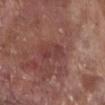Clinical impression:
This lesion was catalogued during total-body skin photography and was not selected for biopsy.
Image and clinical context:
A 15 mm close-up tile from a total-body photography series done for melanoma screening. The patient is a male aged around 70. The lesion is on the right lower leg.